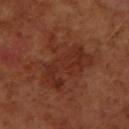Impression: No biopsy was performed on this lesion — it was imaged during a full skin examination and was not determined to be concerning. Image and clinical context: On the left forearm. Captured under cross-polarized illumination. A 15 mm close-up tile from a total-body photography series done for melanoma screening. Approximately 6.5 mm at its widest. A female subject aged 48 to 52.